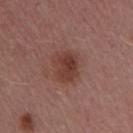This lesion was catalogued during total-body skin photography and was not selected for biopsy.
Automated image analysis of the tile measured an eccentricity of roughly 0.4 and two-axis asymmetry of about 0.15. It also reported a lesion color around L≈39 a*≈23 b*≈24 in CIELAB.
Imaged with white-light lighting.
A female patient aged approximately 45.
The recorded lesion diameter is about 3.5 mm.
A region of skin cropped from a whole-body photographic capture, roughly 15 mm wide.
The lesion is located on the right upper arm.